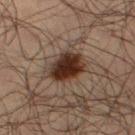| field | value |
|---|---|
| biopsy status | no biopsy performed (imaged during a skin exam) |
| tile lighting | cross-polarized illumination |
| TBP lesion metrics | a lesion area of about 9.5 mm², an outline eccentricity of about 0.55 (0 = round, 1 = elongated), and two-axis asymmetry of about 0.2; a mean CIELAB color near L≈27 a*≈17 b*≈24, a lesion–skin lightness drop of about 16, and a lesion-to-skin contrast of about 15.5 (normalized; higher = more distinct); a border-irregularity index near 2/10, internal color variation of about 4.5 on a 0–10 scale, and a peripheral color-asymmetry measure near 1.5; a nevus-likeness score of about 100/100 and a detector confidence of about 100 out of 100 that the crop contains a lesion |
| imaging modality | 15 mm crop, total-body photography |
| size | about 4 mm |
| patient | male, aged 33–37 |
| site | the left lower leg |